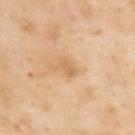Case summary:
- biopsy status: no biopsy performed (imaged during a skin exam)
- location: the upper back
- image: total-body-photography crop, ~15 mm field of view
- illumination: cross-polarized illumination
- subject: male, in their mid- to late 50s
- automated lesion analysis: a detector confidence of about 100 out of 100 that the crop contains a lesion